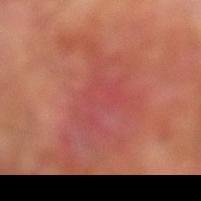Imaged during a routine full-body skin examination; the lesion was not biopsied and no histopathology is available.
The lesion's longest dimension is about 7.5 mm.
The lesion is located on the left lower leg.
This image is a 15 mm lesion crop taken from a total-body photograph.
Imaged with cross-polarized lighting.
The subject is a male aged 68–72.